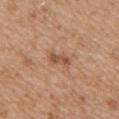biopsy status = no biopsy performed (imaged during a skin exam) | size = ~3 mm (longest diameter) | illumination = white-light | site = the front of the torso | acquisition = total-body-photography crop, ~15 mm field of view | subject = male, in their 50s.